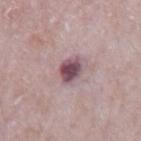Assessment:
Recorded during total-body skin imaging; not selected for excision or biopsy.